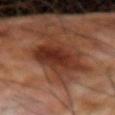biopsy status: no biopsy performed (imaged during a skin exam)
patient: male, approximately 70 years of age
illumination: cross-polarized illumination
body site: the right forearm
automated lesion analysis: a lesion–skin lightness drop of about 12 and a normalized lesion–skin contrast near 10; internal color variation of about 5.5 on a 0–10 scale and radial color variation of about 2; a nevus-likeness score of about 5/100 and a detector confidence of about 100 out of 100 that the crop contains a lesion
image source: ~15 mm tile from a whole-body skin photo